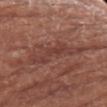Impression: Recorded during total-body skin imaging; not selected for excision or biopsy. Context: The lesion is located on the right forearm. Longest diameter approximately 6.5 mm. A region of skin cropped from a whole-body photographic capture, roughly 15 mm wide. An algorithmic analysis of the crop reported a normalized border contrast of about 5.5. The analysis additionally found border irregularity of about 6.5 on a 0–10 scale, internal color variation of about 3 on a 0–10 scale, and radial color variation of about 1. A female subject, in their mid-70s. The tile uses white-light illumination.